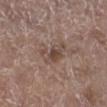<tbp_lesion>
<lighting>white-light</lighting>
<site>leg</site>
<patient>
  <sex>male</sex>
  <age_approx>65</age_approx>
</patient>
<image>
  <source>total-body photography crop</source>
  <field_of_view_mm>15</field_of_view_mm>
</image>
<lesion_size>
  <long_diameter_mm_approx>4.0</long_diameter_mm_approx>
</lesion_size>
</tbp_lesion>